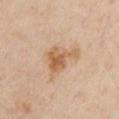{"biopsy_status": "not biopsied; imaged during a skin examination", "patient": {"sex": "male", "age_approx": 65}, "automated_metrics": {"cielab_L": 61, "cielab_a": 18, "cielab_b": 35, "vs_skin_darker_L": 10.0, "vs_skin_contrast_norm": 7.5, "border_irregularity_0_10": 5.5, "peripheral_color_asymmetry": 1.0, "nevus_likeness_0_100": 25, "lesion_detection_confidence_0_100": 100}, "site": "chest", "lesion_size": {"long_diameter_mm_approx": 5.0}, "image": {"source": "total-body photography crop", "field_of_view_mm": 15}, "lighting": "cross-polarized"}